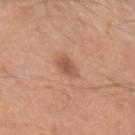The lesion is on the left upper arm. A 15 mm crop from a total-body photograph taken for skin-cancer surveillance. The tile uses white-light illumination. A male patient in their mid-50s. Automated tile analysis of the lesion measured a border-irregularity rating of about 2/10 and a within-lesion color-variation index near 2.5/10. And it measured a nevus-likeness score of about 70/100 and a detector confidence of about 100 out of 100 that the crop contains a lesion. Longest diameter approximately 2.5 mm.